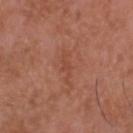Background: The lesion is on the head or neck. A male subject, approximately 50 years of age. The tile uses white-light illumination. Measured at roughly 3.5 mm in maximum diameter. This image is a 15 mm lesion crop taken from a total-body photograph.